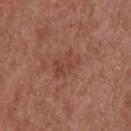  biopsy_status: not biopsied; imaged during a skin examination
  image:
    source: total-body photography crop
    field_of_view_mm: 15
  automated_metrics:
    border_irregularity_0_10: 4.5
    peripheral_color_asymmetry: 0.5
    nevus_likeness_0_100: 0
    lesion_detection_confidence_0_100: 100
  patient:
    sex: female
    age_approx: 60
  site: head or neck
  lesion_size:
    long_diameter_mm_approx: 3.5
  lighting: white-light